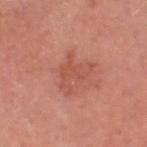Impression: Captured during whole-body skin photography for melanoma surveillance; the lesion was not biopsied. Background: The patient is a male aged 63 to 67. An algorithmic analysis of the crop reported an area of roughly 8 mm², a shape eccentricity near 0.65, and two-axis asymmetry of about 0.6. And it measured a normalized lesion–skin contrast near 5. The software also gave a border-irregularity index near 9/10 and a within-lesion color-variation index near 2.5/10. This image is a 15 mm lesion crop taken from a total-body photograph. On the head or neck. Approximately 4.5 mm at its widest. Captured under white-light illumination.